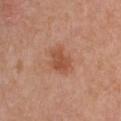This lesion was catalogued during total-body skin photography and was not selected for biopsy. A female subject about 50 years old. On the chest. This is a white-light tile. This image is a 15 mm lesion crop taken from a total-body photograph. Automated tile analysis of the lesion measured a footprint of about 8 mm², an eccentricity of roughly 0.7, and two-axis asymmetry of about 0.3. The software also gave a mean CIELAB color near L≈52 a*≈25 b*≈33, roughly 9 lightness units darker than nearby skin, and a normalized border contrast of about 7. The analysis additionally found border irregularity of about 3 on a 0–10 scale, internal color variation of about 2.5 on a 0–10 scale, and a peripheral color-asymmetry measure near 1. The software also gave a classifier nevus-likeness of about 60/100 and a detector confidence of about 100 out of 100 that the crop contains a lesion.